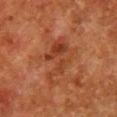workup: catalogued during a skin exam; not biopsied
lighting: cross-polarized
subject: female, approximately 50 years of age
image-analysis metrics: an area of roughly 13 mm², a shape eccentricity near 0.85, and two-axis asymmetry of about 0.4; a lesion color around L≈34 a*≈24 b*≈30 in CIELAB, roughly 7 lightness units darker than nearby skin, and a lesion-to-skin contrast of about 6 (normalized; higher = more distinct); border irregularity of about 6.5 on a 0–10 scale, a color-variation rating of about 5/10, and a peripheral color-asymmetry measure near 1.5
size: ~6 mm (longest diameter)
body site: the chest
image source: ~15 mm crop, total-body skin-cancer survey